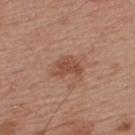Case summary:
- follow-up: catalogued during a skin exam; not biopsied
- size: ~3.5 mm (longest diameter)
- patient: male, approximately 55 years of age
- image source: ~15 mm tile from a whole-body skin photo
- body site: the upper back
- illumination: white-light